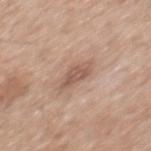  biopsy_status: not biopsied; imaged during a skin examination
  lighting: white-light
  patient:
    sex: male
    age_approx: 60
  lesion_size:
    long_diameter_mm_approx: 3.5
  site: mid back
  image:
    source: total-body photography crop
    field_of_view_mm: 15